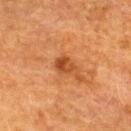No biopsy was performed on this lesion — it was imaged during a full skin examination and was not determined to be concerning.
Longest diameter approximately 2.5 mm.
The subject is a female aged 53 to 57.
On the upper back.
Imaged with cross-polarized lighting.
A roughly 15 mm field-of-view crop from a total-body skin photograph.